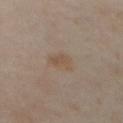The lesion was tiled from a total-body skin photograph and was not biopsied. The patient is a male in their mid- to late 60s. Captured under cross-polarized illumination. A 15 mm close-up extracted from a 3D total-body photography capture. On the abdomen. Longest diameter approximately 3 mm. The lesion-visualizer software estimated a lesion color around L≈50 a*≈13 b*≈27 in CIELAB, a lesion–skin lightness drop of about 5, and a normalized lesion–skin contrast near 5.5.